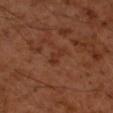| feature | finding |
|---|---|
| biopsy status | imaged on a skin check; not biopsied |
| body site | the right forearm |
| image | total-body-photography crop, ~15 mm field of view |
| patient | male, aged around 60 |